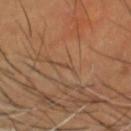workup: no biopsy performed (imaged during a skin exam) | image source: ~15 mm crop, total-body skin-cancer survey | subject: male, in their mid-40s | lighting: cross-polarized | location: the head or neck | lesion size: about 1 mm | automated metrics: a footprint of about 0.5 mm², a shape eccentricity near 0.85, and two-axis asymmetry of about 0.5; a border-irregularity index near 4/10, a within-lesion color-variation index near 0/10, and a peripheral color-asymmetry measure near 0; a nevus-likeness score of about 0/100 and a detector confidence of about 0 out of 100 that the crop contains a lesion.